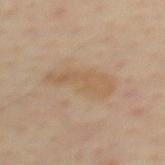follow-up = no biopsy performed (imaged during a skin exam) | diameter = about 7 mm | image source = ~15 mm tile from a whole-body skin photo | automated metrics = a lesion area of about 14 mm², an eccentricity of roughly 0.95, and a symmetry-axis asymmetry near 0.3; border irregularity of about 4.5 on a 0–10 scale and radial color variation of about 0.5; a nevus-likeness score of about 0/100 and a lesion-detection confidence of about 100/100 | lighting = cross-polarized illumination | patient = male, roughly 65 years of age | site = the mid back.